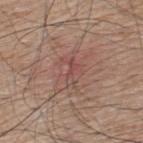The tile uses white-light illumination. A roughly 15 mm field-of-view crop from a total-body skin photograph. The recorded lesion diameter is about 3 mm. The lesion-visualizer software estimated a footprint of about 3 mm². It also reported a border-irregularity index near 5/10, internal color variation of about 0 on a 0–10 scale, and radial color variation of about 0. The lesion is on the back. A male subject about 75 years old.